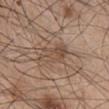image:
  source: total-body photography crop
  field_of_view_mm: 15
lesion_size:
  long_diameter_mm_approx: 5.0
patient:
  sex: male
  age_approx: 45
site: chest